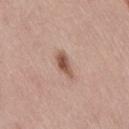  lighting: white-light
  patient:
    sex: female
    age_approx: 40
  image:
    source: total-body photography crop
    field_of_view_mm: 15
  site: right thigh
  lesion_size:
    long_diameter_mm_approx: 3.5